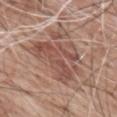Assessment:
This lesion was catalogued during total-body skin photography and was not selected for biopsy.
Context:
The lesion is located on the mid back. A close-up tile cropped from a whole-body skin photograph, about 15 mm across. The total-body-photography lesion software estimated a shape eccentricity near 0.9 and a shape-asymmetry score of about 0.4 (0 = symmetric). It also reported a peripheral color-asymmetry measure near 2. Imaged with white-light lighting. The recorded lesion diameter is about 9 mm. The subject is a male approximately 60 years of age.